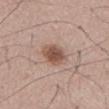Part of a total-body skin-imaging series; this lesion was reviewed on a skin check and was not flagged for biopsy. Longest diameter approximately 3 mm. The patient is a male approximately 55 years of age. A 15 mm crop from a total-body photograph taken for skin-cancer surveillance. The total-body-photography lesion software estimated a footprint of about 7 mm², an eccentricity of roughly 0.55, and two-axis asymmetry of about 0.2. The analysis additionally found border irregularity of about 1.5 on a 0–10 scale, internal color variation of about 3.5 on a 0–10 scale, and a peripheral color-asymmetry measure near 1. It also reported a nevus-likeness score of about 95/100 and lesion-presence confidence of about 100/100. The tile uses white-light illumination.